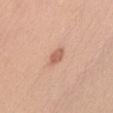Findings:
* notes: no biopsy performed (imaged during a skin exam)
* image source: ~15 mm crop, total-body skin-cancer survey
* TBP lesion metrics: an average lesion color of about L≈61 a*≈24 b*≈31 (CIELAB), roughly 11 lightness units darker than nearby skin, and a lesion-to-skin contrast of about 7 (normalized; higher = more distinct); a border-irregularity rating of about 3/10 and a within-lesion color-variation index near 1.5/10
* subject: female, in their mid-20s
* diameter: ~3 mm (longest diameter)
* lighting: white-light illumination
* anatomic site: the head or neck